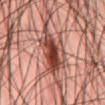Part of a total-body skin-imaging series; this lesion was reviewed on a skin check and was not flagged for biopsy.
The lesion-visualizer software estimated an eccentricity of roughly 0.85 and a symmetry-axis asymmetry near 0.4. And it measured a mean CIELAB color near L≈43 a*≈25 b*≈26, roughly 16 lightness units darker than nearby skin, and a lesion-to-skin contrast of about 11.5 (normalized; higher = more distinct).
A 15 mm close-up extracted from a 3D total-body photography capture.
The lesion's longest dimension is about 7 mm.
Captured under cross-polarized illumination.
A male subject, aged 43–47.
Located on the abdomen.